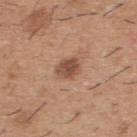Context: Cropped from a whole-body photographic skin survey; the tile spans about 15 mm. From the back. This is a white-light tile. A male subject, aged 38–42. Longest diameter approximately 2.5 mm.